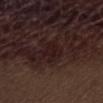Impression: Part of a total-body skin-imaging series; this lesion was reviewed on a skin check and was not flagged for biopsy. Background: From the abdomen. A male patient aged 68 to 72. The total-body-photography lesion software estimated a mean CIELAB color near L≈17 a*≈16 b*≈14 and about 5 CIELAB-L* units darker than the surrounding skin. The analysis additionally found a color-variation rating of about 2/10 and radial color variation of about 0.5. It also reported a classifier nevus-likeness of about 0/100. Imaged with white-light lighting. This image is a 15 mm lesion crop taken from a total-body photograph.